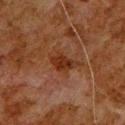Clinical impression: The lesion was photographed on a routine skin check and not biopsied; there is no pathology result. Image and clinical context: Automated tile analysis of the lesion measured a footprint of about 6.5 mm². The analysis additionally found a lesion color around L≈25 a*≈20 b*≈27 in CIELAB and roughly 7 lightness units darker than nearby skin. And it measured a border-irregularity rating of about 4/10, internal color variation of about 2.5 on a 0–10 scale, and peripheral color asymmetry of about 1. This is a cross-polarized tile. A roughly 15 mm field-of-view crop from a total-body skin photograph. A male patient, aged 78–82. The lesion's longest dimension is about 4 mm. The lesion is on the back.